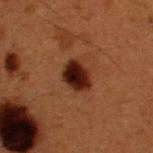| field | value |
|---|---|
| workup | catalogued during a skin exam; not biopsied |
| patient | male, aged around 50 |
| imaging modality | ~15 mm crop, total-body skin-cancer survey |
| illumination | cross-polarized |
| lesion diameter | about 3.5 mm |
| location | the upper back |